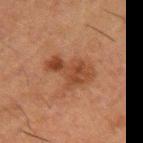Captured during whole-body skin photography for melanoma surveillance; the lesion was not biopsied. The lesion's longest dimension is about 5 mm. The subject is a male aged approximately 50. Imaged with cross-polarized lighting. A roughly 15 mm field-of-view crop from a total-body skin photograph. Located on the left upper arm.